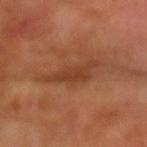Imaged during a routine full-body skin examination; the lesion was not biopsied and no histopathology is available.
The patient is a male aged approximately 65.
A close-up tile cropped from a whole-body skin photograph, about 15 mm across.